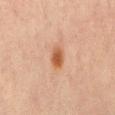notes = catalogued during a skin exam; not biopsied | subject = male, approximately 70 years of age | illumination = cross-polarized | imaging modality = 15 mm crop, total-body photography | site = the abdomen.